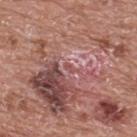Image and clinical context:
A 15 mm crop from a total-body photograph taken for skin-cancer surveillance. The patient is a male in their 70s. This is a white-light tile. Located on the back. Measured at roughly 1.5 mm in maximum diameter. Automated tile analysis of the lesion measured a lesion area of about 1 mm², a shape eccentricity near 0.85, and two-axis asymmetry of about 0.35.
Diagnosis:
Histopathologically confirmed as a melanoma in situ.